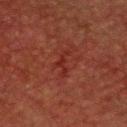size = ~3.5 mm (longest diameter)
patient = male, in their 60s
site = the head or neck
image = 15 mm crop, total-body photography
tile lighting = cross-polarized illumination
automated metrics = border irregularity of about 9.5 on a 0–10 scale, internal color variation of about 0 on a 0–10 scale, and a peripheral color-asymmetry measure near 0; a classifier nevus-likeness of about 0/100 and a detector confidence of about 80 out of 100 that the crop contains a lesion